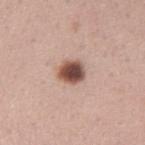biopsy_status: not biopsied; imaged during a skin examination
site: left forearm
automated_metrics:
  cielab_L: 49
  cielab_a: 21
  cielab_b: 25
  vs_skin_darker_L: 20.0
  vs_skin_contrast_norm: 13.0
  border_irregularity_0_10: 1.5
  color_variation_0_10: 5.5
  peripheral_color_asymmetry: 1.5
lesion_size:
  long_diameter_mm_approx: 3.0
image:
  source: total-body photography crop
  field_of_view_mm: 15
patient:
  sex: female
  age_approx: 30
lighting: white-light